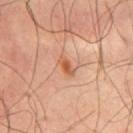biopsy_status: not biopsied; imaged during a skin examination
lesion_size:
  long_diameter_mm_approx: 2.5
patient:
  sex: male
  age_approx: 55
lighting: cross-polarized
image:
  source: total-body photography crop
  field_of_view_mm: 15
site: chest